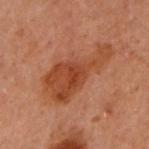The lesion was photographed on a routine skin check and not biopsied; there is no pathology result.
Located on the left arm.
A female subject, aged approximately 60.
The tile uses cross-polarized illumination.
A lesion tile, about 15 mm wide, cut from a 3D total-body photograph.